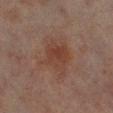Clinical impression:
The lesion was photographed on a routine skin check and not biopsied; there is no pathology result.